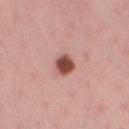<tbp_lesion>
  <biopsy_status>not biopsied; imaged during a skin examination</biopsy_status>
  <patient>
    <sex>female</sex>
    <age_approx>35</age_approx>
  </patient>
  <image>
    <source>total-body photography crop</source>
    <field_of_view_mm>15</field_of_view_mm>
  </image>
  <site>right thigh</site>
</tbp_lesion>